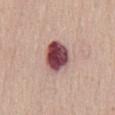follow-up: imaged on a skin check; not biopsied
illumination: white-light
image source: total-body-photography crop, ~15 mm field of view
automated lesion analysis: an automated nevus-likeness rating near 0 out of 100 and a lesion-detection confidence of about 100/100
patient: male, in their mid- to late 60s
location: the chest